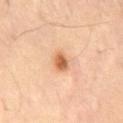workup = total-body-photography surveillance lesion; no biopsy
image = ~15 mm tile from a whole-body skin photo
lighting = cross-polarized
size = ≈2.5 mm
subject = male, approximately 55 years of age
anatomic site = the mid back